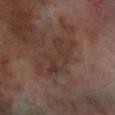The lesion was photographed on a routine skin check and not biopsied; there is no pathology result. On the leg. A lesion tile, about 15 mm wide, cut from a 3D total-body photograph. Measured at roughly 5.5 mm in maximum diameter. Imaged with cross-polarized lighting. The total-body-photography lesion software estimated an area of roughly 10 mm², an eccentricity of roughly 0.85, and a shape-asymmetry score of about 0.5 (0 = symmetric). The analysis additionally found a within-lesion color-variation index near 4.5/10 and radial color variation of about 1.5. The analysis additionally found lesion-presence confidence of about 100/100. A male subject, aged approximately 70.